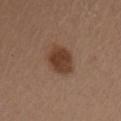workup: imaged on a skin check; not biopsied
image: 15 mm crop, total-body photography
location: the front of the torso
subject: female, roughly 40 years of age
lesion diameter: ~4 mm (longest diameter)